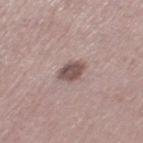Clinical impression:
Captured during whole-body skin photography for melanoma surveillance; the lesion was not biopsied.
Clinical summary:
A 15 mm close-up extracted from a 3D total-body photography capture. A female subject, roughly 40 years of age. Located on the left thigh.